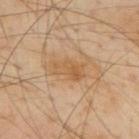The lesion was tiled from a total-body skin photograph and was not biopsied. A lesion tile, about 15 mm wide, cut from a 3D total-body photograph. On the mid back. The subject is a male aged approximately 55.